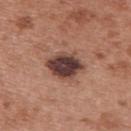* notes · catalogued during a skin exam; not biopsied
* image source · ~15 mm tile from a whole-body skin photo
* site · the upper back
* subject · female, about 40 years old
* lighting · white-light